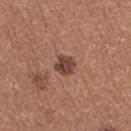{"biopsy_status": "not biopsied; imaged during a skin examination", "site": "right thigh", "image": {"source": "total-body photography crop", "field_of_view_mm": 15}, "lighting": "white-light", "patient": {"sex": "female", "age_approx": 35}, "lesion_size": {"long_diameter_mm_approx": 2.5}, "automated_metrics": {"cielab_L": 43, "cielab_a": 21, "cielab_b": 25, "vs_skin_darker_L": 13.0, "border_irregularity_0_10": 2.0}}